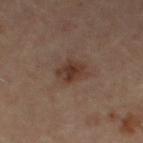notes: total-body-photography surveillance lesion; no biopsy
lesion size: ~3.5 mm (longest diameter)
subject: female, about 35 years old
anatomic site: the right thigh
automated metrics: a shape eccentricity near 0.75 and a shape-asymmetry score of about 0.2 (0 = symmetric); about 9 CIELAB-L* units darker than the surrounding skin and a normalized border contrast of about 8; border irregularity of about 2.5 on a 0–10 scale and a peripheral color-asymmetry measure near 1
image source: ~15 mm crop, total-body skin-cancer survey